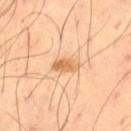Recorded during total-body skin imaging; not selected for excision or biopsy.
Imaged with cross-polarized lighting.
A roughly 15 mm field-of-view crop from a total-body skin photograph.
A male patient in their mid-50s.
About 3 mm across.
The lesion is located on the right thigh.